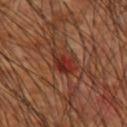Q: Is there a histopathology result?
A: catalogued during a skin exam; not biopsied
Q: Patient demographics?
A: male, aged approximately 60
Q: Where on the body is the lesion?
A: the right upper arm
Q: How large is the lesion?
A: about 4 mm
Q: How was this image acquired?
A: ~15 mm tile from a whole-body skin photo
Q: What did automated image analysis measure?
A: a border-irregularity rating of about 4/10, internal color variation of about 7 on a 0–10 scale, and radial color variation of about 2.5; an automated nevus-likeness rating near 5 out of 100
Q: How was the tile lit?
A: cross-polarized